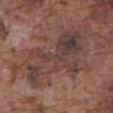Assessment:
Imaged during a routine full-body skin examination; the lesion was not biopsied and no histopathology is available.
Background:
The subject is a male aged 73–77. A lesion tile, about 15 mm wide, cut from a 3D total-body photograph. The lesion is located on the abdomen.